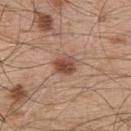  biopsy_status: not biopsied; imaged during a skin examination
  patient:
    sex: male
    age_approx: 50
  site: upper back
  automated_metrics:
    area_mm2_approx: 6.0
    eccentricity: 0.45
    shape_asymmetry: 0.15
    color_variation_0_10: 6.0
    peripheral_color_asymmetry: 2.0
  lighting: white-light
  image:
    source: total-body photography crop
    field_of_view_mm: 15